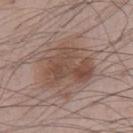Assessment:
This lesion was catalogued during total-body skin photography and was not selected for biopsy.
Context:
Located on the front of the torso. A roughly 15 mm field-of-view crop from a total-body skin photograph. A male patient, aged approximately 65. An algorithmic analysis of the crop reported a lesion area of about 23 mm², an outline eccentricity of about 0.6 (0 = round, 1 = elongated), and a symmetry-axis asymmetry near 0.35. The software also gave a border-irregularity rating of about 4.5/10, internal color variation of about 5 on a 0–10 scale, and peripheral color asymmetry of about 2. Measured at roughly 6.5 mm in maximum diameter.